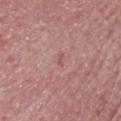Q: Was a biopsy performed?
A: catalogued during a skin exam; not biopsied
Q: Patient demographics?
A: male, about 85 years old or older
Q: What is the anatomic site?
A: the head or neck
Q: How was this image acquired?
A: total-body-photography crop, ~15 mm field of view